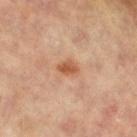Part of a total-body skin-imaging series; this lesion was reviewed on a skin check and was not flagged for biopsy.
Approximately 2.5 mm at its widest.
From the left leg.
Automated tile analysis of the lesion measured a lesion area of about 3.5 mm² and a symmetry-axis asymmetry near 0.25.
A region of skin cropped from a whole-body photographic capture, roughly 15 mm wide.
This is a cross-polarized tile.
A female subject, aged 63 to 67.